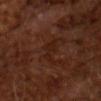Part of a total-body skin-imaging series; this lesion was reviewed on a skin check and was not flagged for biopsy. Measured at roughly 4.5 mm in maximum diameter. Automated tile analysis of the lesion measured a lesion color around L≈20 a*≈19 b*≈24 in CIELAB, about 4 CIELAB-L* units darker than the surrounding skin, and a normalized border contrast of about 5. A lesion tile, about 15 mm wide, cut from a 3D total-body photograph. Imaged with cross-polarized lighting. The patient is a male roughly 65 years of age.